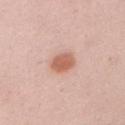Measured at roughly 3 mm in maximum diameter. The lesion is located on the left upper arm. A male patient, about 35 years old. A close-up tile cropped from a whole-body skin photograph, about 15 mm across.